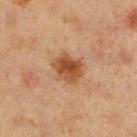biopsy status: total-body-photography surveillance lesion; no biopsy
acquisition: ~15 mm tile from a whole-body skin photo
illumination: cross-polarized illumination
site: the right thigh
patient: female, aged around 40
TBP lesion metrics: an eccentricity of roughly 0.45 and a shape-asymmetry score of about 0.2 (0 = symmetric); a nevus-likeness score of about 95/100 and a lesion-detection confidence of about 100/100
lesion size: ~3.5 mm (longest diameter)